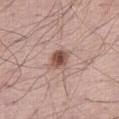<lesion>
  <biopsy_status>not biopsied; imaged during a skin examination</biopsy_status>
  <site>right thigh</site>
  <image>
    <source>total-body photography crop</source>
    <field_of_view_mm>15</field_of_view_mm>
  </image>
  <patient>
    <sex>male</sex>
    <age_approx>55</age_approx>
  </patient>
  <lighting>white-light</lighting>
  <lesion_size>
    <long_diameter_mm_approx>2.5</long_diameter_mm_approx>
  </lesion_size>
</lesion>